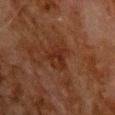Part of a total-body skin-imaging series; this lesion was reviewed on a skin check and was not flagged for biopsy. The lesion is located on the upper back. About 3.5 mm across. A male patient, aged 78–82. A 15 mm close-up tile from a total-body photography series done for melanoma screening. The lesion-visualizer software estimated a within-lesion color-variation index near 3.5/10 and peripheral color asymmetry of about 1. It also reported a nevus-likeness score of about 10/100 and a lesion-detection confidence of about 100/100. Imaged with cross-polarized lighting.